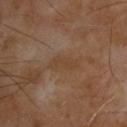Recorded during total-body skin imaging; not selected for excision or biopsy. The tile uses cross-polarized illumination. A roughly 15 mm field-of-view crop from a total-body skin photograph. An algorithmic analysis of the crop reported an average lesion color of about L≈37 a*≈15 b*≈29 (CIELAB), about 5 CIELAB-L* units darker than the surrounding skin, and a normalized border contrast of about 6. On the upper back. A male subject, aged 53–57.